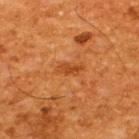workup=total-body-photography surveillance lesion; no biopsy | body site=the upper back | illumination=cross-polarized illumination | imaging modality=15 mm crop, total-body photography | subject=male, aged 63–67 | TBP lesion metrics=a border-irregularity index near 4/10, internal color variation of about 0.5 on a 0–10 scale, and a peripheral color-asymmetry measure near 0; a classifier nevus-likeness of about 5/100 and lesion-presence confidence of about 100/100 | lesion diameter=~3 mm (longest diameter).